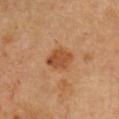{"biopsy_status": "not biopsied; imaged during a skin examination", "lighting": "cross-polarized", "automated_metrics": {"area_mm2_approx": 7.0, "eccentricity": 0.55, "cielab_L": 49, "cielab_a": 25, "cielab_b": 38, "vs_skin_darker_L": 10.0}, "patient": {"sex": "male", "age_approx": 50}, "site": "chest", "image": {"source": "total-body photography crop", "field_of_view_mm": 15}}